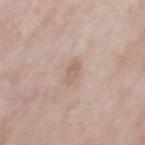This lesion was catalogued during total-body skin photography and was not selected for biopsy.
This image is a 15 mm lesion crop taken from a total-body photograph.
The lesion is located on the mid back.
A male subject aged 53–57.